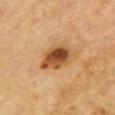{"biopsy_status": "not biopsied; imaged during a skin examination", "automated_metrics": {"border_irregularity_0_10": 2.5, "color_variation_0_10": 6.5, "nevus_likeness_0_100": 90}, "lighting": "cross-polarized", "patient": {"sex": "male", "age_approx": "85+"}, "image": {"source": "total-body photography crop", "field_of_view_mm": 15}, "lesion_size": {"long_diameter_mm_approx": 4.5}, "site": "chest"}